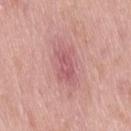workup: catalogued during a skin exam; not biopsied
body site: the leg
patient: male, aged 53–57
illumination: white-light illumination
TBP lesion metrics: border irregularity of about 3.5 on a 0–10 scale, a within-lesion color-variation index near 2.5/10, and peripheral color asymmetry of about 1; a nevus-likeness score of about 0/100 and a lesion-detection confidence of about 100/100
acquisition: ~15 mm tile from a whole-body skin photo
lesion size: about 4.5 mm